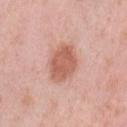follow-up: total-body-photography surveillance lesion; no biopsy | tile lighting: white-light illumination | automated lesion analysis: a lesion area of about 12 mm², an outline eccentricity of about 0.7 (0 = round, 1 = elongated), and a shape-asymmetry score of about 0.15 (0 = symmetric); a normalized lesion–skin contrast near 8; border irregularity of about 1.5 on a 0–10 scale and peripheral color asymmetry of about 1; lesion-presence confidence of about 100/100 | body site: the left thigh | diameter: about 5 mm | subject: female, aged around 40 | image source: ~15 mm tile from a whole-body skin photo.